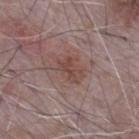Notes:
- subject: male, approximately 65 years of age
- site: the back
- imaging modality: ~15 mm tile from a whole-body skin photo